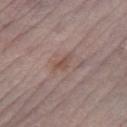biopsy status — total-body-photography surveillance lesion; no biopsy | image-analysis metrics — a mean CIELAB color near L≈50 a*≈18 b*≈24 | image source — 15 mm crop, total-body photography | subject — male, roughly 75 years of age | location — the leg | tile lighting — white-light illumination | lesion size — ≈2.5 mm.